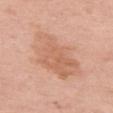Findings:
* workup: catalogued during a skin exam; not biopsied